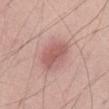notes: imaged on a skin check; not biopsied
subject: male, about 55 years old
image: ~15 mm tile from a whole-body skin photo
lighting: white-light illumination
anatomic site: the abdomen
image-analysis metrics: a footprint of about 9 mm² and a shape-asymmetry score of about 0.2 (0 = symmetric); a mean CIELAB color near L≈57 a*≈24 b*≈23, about 10 CIELAB-L* units darker than the surrounding skin, and a lesion-to-skin contrast of about 6.5 (normalized; higher = more distinct)
lesion size: ~4 mm (longest diameter)